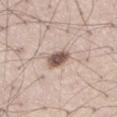Clinical impression:
Part of a total-body skin-imaging series; this lesion was reviewed on a skin check and was not flagged for biopsy.
Context:
Approximately 3 mm at its widest. The lesion is located on the right thigh. The tile uses white-light illumination. A lesion tile, about 15 mm wide, cut from a 3D total-body photograph. A male subject, aged 38 to 42.